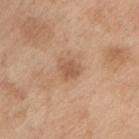{"biopsy_status": "not biopsied; imaged during a skin examination", "patient": {"sex": "male", "age_approx": 70}, "lighting": "white-light", "image": {"source": "total-body photography crop", "field_of_view_mm": 15}, "lesion_size": {"long_diameter_mm_approx": 2.5}, "site": "right upper arm"}